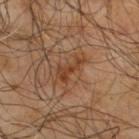Impression: No biopsy was performed on this lesion — it was imaged during a full skin examination and was not determined to be concerning. Context: Approximately 3.5 mm at its widest. The tile uses cross-polarized illumination. A roughly 15 mm field-of-view crop from a total-body skin photograph. A male subject, aged approximately 65. From the upper back. The lesion-visualizer software estimated a border-irregularity rating of about 6/10 and a within-lesion color-variation index near 1.5/10. And it measured a nevus-likeness score of about 0/100.